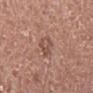No biopsy was performed on this lesion — it was imaged during a full skin examination and was not determined to be concerning.
From the right lower leg.
Cropped from a whole-body photographic skin survey; the tile spans about 15 mm.
The subject is a female aged 48–52.